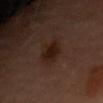Context:
A 15 mm crop from a total-body photograph taken for skin-cancer surveillance. A female patient, approximately 60 years of age. From the arm. Approximately 4.5 mm at its widest.